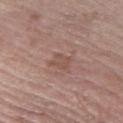workup: imaged on a skin check; not biopsied | patient: male, about 60 years old | location: the left thigh | lighting: white-light illumination | lesion size: ≈2.5 mm | image: ~15 mm crop, total-body skin-cancer survey | automated lesion analysis: a classifier nevus-likeness of about 0/100 and lesion-presence confidence of about 100/100.